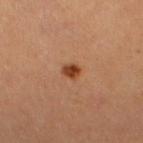Image and clinical context:
A region of skin cropped from a whole-body photographic capture, roughly 15 mm wide. On the left lower leg. Approximately 2 mm at its widest. The subject is a female aged approximately 25. The total-body-photography lesion software estimated a footprint of about 3 mm², a shape eccentricity near 0.6, and two-axis asymmetry of about 0.2. It also reported an average lesion color of about L≈42 a*≈25 b*≈35 (CIELAB), a lesion–skin lightness drop of about 13, and a normalized lesion–skin contrast near 10.5. The analysis additionally found a border-irregularity index near 1.5/10 and a within-lesion color-variation index near 3/10.